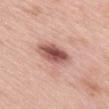Assessment: The lesion was photographed on a routine skin check and not biopsied; there is no pathology result. Context: A close-up tile cropped from a whole-body skin photograph, about 15 mm across. The tile uses white-light illumination. The lesion is on the mid back. The patient is a female aged 63–67.